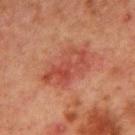Part of a total-body skin-imaging series; this lesion was reviewed on a skin check and was not flagged for biopsy. A male patient aged approximately 65. Imaged with cross-polarized lighting. Approximately 6 mm at its widest. A roughly 15 mm field-of-view crop from a total-body skin photograph. The lesion-visualizer software estimated an area of roughly 15 mm² and a shape-asymmetry score of about 0.25 (0 = symmetric). It also reported a mean CIELAB color near L≈39 a*≈25 b*≈27 and roughly 7 lightness units darker than nearby skin. The lesion is located on the chest.